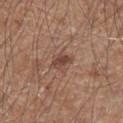- acquisition: total-body-photography crop, ~15 mm field of view
- patient: male, aged around 60
- lighting: white-light illumination
- body site: the arm
- size: ~2.5 mm (longest diameter)